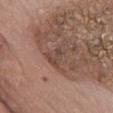Q: Where on the body is the lesion?
A: the front of the torso
Q: What is the imaging modality?
A: total-body-photography crop, ~15 mm field of view
Q: What lighting was used for the tile?
A: white-light illumination
Q: Patient demographics?
A: male, about 75 years old